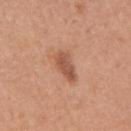biopsy_status: not biopsied; imaged during a skin examination
patient:
  sex: female
  age_approx: 35
image:
  source: total-body photography crop
  field_of_view_mm: 15
site: right upper arm